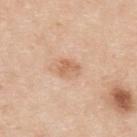Recorded during total-body skin imaging; not selected for excision or biopsy.
On the upper back.
A 15 mm crop from a total-body photograph taken for skin-cancer surveillance.
The patient is a male roughly 35 years of age.